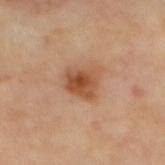Captured during whole-body skin photography for melanoma surveillance; the lesion was not biopsied. On the mid back. A lesion tile, about 15 mm wide, cut from a 3D total-body photograph. The lesion-visualizer software estimated a footprint of about 9 mm², a shape eccentricity near 0.5, and a symmetry-axis asymmetry near 0.25. The software also gave a mean CIELAB color near L≈52 a*≈24 b*≈36. It also reported a classifier nevus-likeness of about 90/100 and a lesion-detection confidence of about 100/100. Captured under cross-polarized illumination.